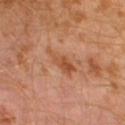Context:
The lesion is located on the left lower leg. A male patient about 30 years old. Cropped from a total-body skin-imaging series; the visible field is about 15 mm.